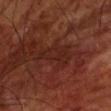Assessment:
The lesion was photographed on a routine skin check and not biopsied; there is no pathology result.
Context:
Located on the left forearm. The tile uses cross-polarized illumination. Approximately 4.5 mm at its widest. A region of skin cropped from a whole-body photographic capture, roughly 15 mm wide. A male subject, aged approximately 70.